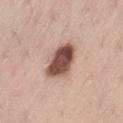biopsy status = imaged on a skin check; not biopsied
acquisition = 15 mm crop, total-body photography
site = the left thigh
image-analysis metrics = a mean CIELAB color near L≈51 a*≈20 b*≈25, roughly 20 lightness units darker than nearby skin, and a normalized lesion–skin contrast near 12.5; a color-variation rating of about 6/10 and a peripheral color-asymmetry measure near 2
subject = male, aged approximately 40
lighting = white-light illumination